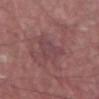{
  "biopsy_status": "not biopsied; imaged during a skin examination",
  "site": "abdomen",
  "lighting": "white-light",
  "image": {
    "source": "total-body photography crop",
    "field_of_view_mm": 15
  },
  "patient": {
    "sex": "male",
    "age_approx": 40
  },
  "lesion_size": {
    "long_diameter_mm_approx": 4.0
  }
}